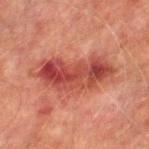<tbp_lesion>
<biopsy_status>not biopsied; imaged during a skin examination</biopsy_status>
<lighting>cross-polarized</lighting>
<image>
  <source>total-body photography crop</source>
  <field_of_view_mm>15</field_of_view_mm>
</image>
<lesion_size>
  <long_diameter_mm_approx>9.0</long_diameter_mm_approx>
</lesion_size>
<patient>
  <sex>male</sex>
  <age_approx>75</age_approx>
</patient>
<site>leg</site>
</tbp_lesion>